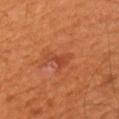This lesion was catalogued during total-body skin photography and was not selected for biopsy. A male patient, aged around 55. A lesion tile, about 15 mm wide, cut from a 3D total-body photograph. The lesion is on the right upper arm. The total-body-photography lesion software estimated a border-irregularity index near 6/10, internal color variation of about 1.5 on a 0–10 scale, and a peripheral color-asymmetry measure near 0.5. And it measured a lesion-detection confidence of about 100/100.